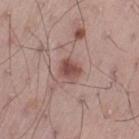Captured during whole-body skin photography for melanoma surveillance; the lesion was not biopsied. A roughly 15 mm field-of-view crop from a total-body skin photograph. Captured under white-light illumination. A male patient, approximately 50 years of age. The lesion is on the leg. An algorithmic analysis of the crop reported a mean CIELAB color near L≈48 a*≈22 b*≈23 and about 12 CIELAB-L* units darker than the surrounding skin. The analysis additionally found a border-irregularity rating of about 2/10, a within-lesion color-variation index near 2.5/10, and peripheral color asymmetry of about 1.